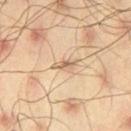The recorded lesion diameter is about 3 mm. A male patient in their mid-40s. A 15 mm close-up extracted from a 3D total-body photography capture. Located on the left thigh. Automated tile analysis of the lesion measured an area of roughly 3.5 mm², a shape eccentricity near 0.9, and two-axis asymmetry of about 0.3. It also reported an average lesion color of about L≈65 a*≈15 b*≈32 (CIELAB), a lesion–skin lightness drop of about 11, and a normalized border contrast of about 6.5.